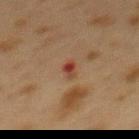This lesion was catalogued during total-body skin photography and was not selected for biopsy. The subject is a female about 40 years old. Automated image analysis of the tile measured a lesion color around L≈34 a*≈22 b*≈26 in CIELAB, about 9 CIELAB-L* units darker than the surrounding skin, and a lesion-to-skin contrast of about 8.5 (normalized; higher = more distinct). And it measured a color-variation rating of about 1/10 and radial color variation of about 0. The lesion is on the back. A close-up tile cropped from a whole-body skin photograph, about 15 mm across.